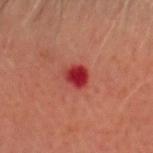Notes:
– follow-up — no biopsy performed (imaged during a skin exam)
– lesion diameter — ~2.5 mm (longest diameter)
– site — the head or neck
– patient — male, aged 43–47
– image source — 15 mm crop, total-body photography